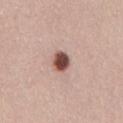Q: Is there a histopathology result?
A: imaged on a skin check; not biopsied
Q: Lesion size?
A: about 2.5 mm
Q: What lighting was used for the tile?
A: white-light
Q: Where on the body is the lesion?
A: the back
Q: What is the imaging modality?
A: total-body-photography crop, ~15 mm field of view
Q: What are the patient's age and sex?
A: female, in their mid- to late 50s
Q: What did automated image analysis measure?
A: a lesion color around L≈49 a*≈22 b*≈24 in CIELAB, about 20 CIELAB-L* units darker than the surrounding skin, and a normalized border contrast of about 13; border irregularity of about 1 on a 0–10 scale, a color-variation rating of about 4/10, and peripheral color asymmetry of about 1; a classifier nevus-likeness of about 100/100 and a lesion-detection confidence of about 100/100